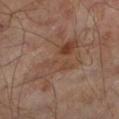– biopsy status · no biopsy performed (imaged during a skin exam)
– lighting · cross-polarized illumination
– image-analysis metrics · an eccentricity of roughly 0.8 and a shape-asymmetry score of about 0.6 (0 = symmetric); a color-variation rating of about 7.5/10; a nevus-likeness score of about 0/100 and lesion-presence confidence of about 100/100
– size · about 8 mm
– image source · total-body-photography crop, ~15 mm field of view
– site · the left lower leg
– patient · male, aged approximately 65